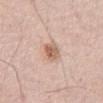• notes — total-body-photography surveillance lesion; no biopsy
• patient — male, in their mid-50s
• body site — the abdomen
• automated lesion analysis — a mean CIELAB color near L≈61 a*≈20 b*≈29 and a lesion–skin lightness drop of about 12; a border-irregularity rating of about 2/10, a color-variation rating of about 4/10, and a peripheral color-asymmetry measure near 1.5; an automated nevus-likeness rating near 85 out of 100 and a lesion-detection confidence of about 100/100
• illumination — white-light
• image source — ~15 mm crop, total-body skin-cancer survey
• size — ~3 mm (longest diameter)